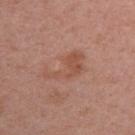Recorded during total-body skin imaging; not selected for excision or biopsy. Approximately 5.5 mm at its widest. A female subject, aged approximately 55. Automated tile analysis of the lesion measured about 6 CIELAB-L* units darker than the surrounding skin and a lesion-to-skin contrast of about 5.5 (normalized; higher = more distinct). The software also gave a border-irregularity index near 7/10, a color-variation rating of about 2.5/10, and a peripheral color-asymmetry measure near 1. It also reported an automated nevus-likeness rating near 5 out of 100 and lesion-presence confidence of about 100/100. On the right upper arm. A 15 mm close-up extracted from a 3D total-body photography capture.